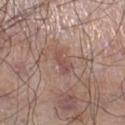Assessment: Recorded during total-body skin imaging; not selected for excision or biopsy. Acquisition and patient details: This is a white-light tile. A male patient, in their 70s. A 15 mm close-up tile from a total-body photography series done for melanoma screening. An algorithmic analysis of the crop reported a lesion area of about 3 mm² and a symmetry-axis asymmetry near 0.3. The analysis additionally found a mean CIELAB color near L≈50 a*≈21 b*≈23 and a lesion-to-skin contrast of about 5.5 (normalized; higher = more distinct). The analysis additionally found a border-irregularity index near 3/10, internal color variation of about 1 on a 0–10 scale, and a peripheral color-asymmetry measure near 0. On the right lower leg. The lesion's longest dimension is about 3 mm.